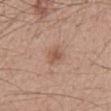The lesion was photographed on a routine skin check and not biopsied; there is no pathology result. A 15 mm close-up tile from a total-body photography series done for melanoma screening. The patient is a male aged 48 to 52. Located on the mid back.